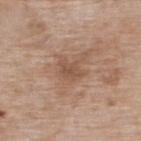{"biopsy_status": "not biopsied; imaged during a skin examination", "automated_metrics": {"border_irregularity_0_10": 3.5, "color_variation_0_10": 2.0, "peripheral_color_asymmetry": 0.5, "nevus_likeness_0_100": 0, "lesion_detection_confidence_0_100": 100}, "image": {"source": "total-body photography crop", "field_of_view_mm": 15}, "lesion_size": {"long_diameter_mm_approx": 3.0}, "site": "upper back", "lighting": "white-light", "patient": {"sex": "female", "age_approx": 75}}